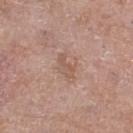The lesion was tiled from a total-body skin photograph and was not biopsied.
The subject is a female in their mid-60s.
On the right thigh.
Imaged with white-light lighting.
A 15 mm close-up extracted from a 3D total-body photography capture.